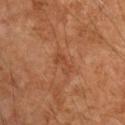workup: total-body-photography surveillance lesion; no biopsy | site: the right upper arm | tile lighting: cross-polarized illumination | lesion size: ~3 mm (longest diameter) | imaging modality: total-body-photography crop, ~15 mm field of view | subject: male, in their mid-50s | image-analysis metrics: an area of roughly 3 mm² and a shape eccentricity near 0.9; border irregularity of about 6 on a 0–10 scale, a color-variation rating of about 1/10, and peripheral color asymmetry of about 0.5; an automated nevus-likeness rating near 0 out of 100.